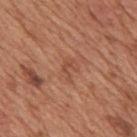notes: catalogued during a skin exam; not biopsied